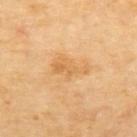This lesion was catalogued during total-body skin photography and was not selected for biopsy. A female subject aged approximately 70. Automated image analysis of the tile measured an average lesion color of about L≈68 a*≈22 b*≈47 (CIELAB), a lesion–skin lightness drop of about 8, and a normalized lesion–skin contrast near 5.5. A lesion tile, about 15 mm wide, cut from a 3D total-body photograph. This is a cross-polarized tile. Longest diameter approximately 4 mm. The lesion is on the upper back.